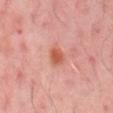| key | value |
|---|---|
| follow-up | total-body-photography surveillance lesion; no biopsy |
| illumination | cross-polarized illumination |
| automated lesion analysis | a lesion color around L≈56 a*≈30 b*≈34 in CIELAB, about 10 CIELAB-L* units darker than the surrounding skin, and a lesion-to-skin contrast of about 9 (normalized; higher = more distinct) |
| anatomic site | the mid back |
| lesion diameter | about 2.5 mm |
| patient | male, in their 50s |
| imaging modality | total-body-photography crop, ~15 mm field of view |